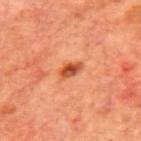• biopsy status · catalogued during a skin exam; not biopsied
• imaging modality · total-body-photography crop, ~15 mm field of view
• patient · male, aged approximately 70
• anatomic site · the mid back